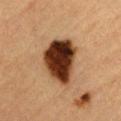Q: Was a biopsy performed?
A: imaged on a skin check; not biopsied
Q: What lighting was used for the tile?
A: cross-polarized
Q: Automated lesion metrics?
A: a footprint of about 20 mm² and a symmetry-axis asymmetry near 0.25; a border-irregularity rating of about 2/10 and peripheral color asymmetry of about 2.5; a nevus-likeness score of about 100/100 and lesion-presence confidence of about 100/100
Q: What is the lesion's diameter?
A: ≈6 mm
Q: What are the patient's age and sex?
A: male, aged around 85 or older
Q: What kind of image is this?
A: ~15 mm tile from a whole-body skin photo
Q: Where on the body is the lesion?
A: the abdomen